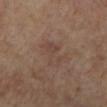No biopsy was performed on this lesion — it was imaged during a full skin examination and was not determined to be concerning. A female patient aged 63–67. Cropped from a whole-body photographic skin survey; the tile spans about 15 mm. The lesion is on the leg.